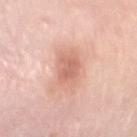biopsy status = catalogued during a skin exam; not biopsied | site = the chest | illumination = white-light | lesion size = ~4.5 mm (longest diameter) | automated lesion analysis = a lesion area of about 9.5 mm², a shape eccentricity near 0.8, and a symmetry-axis asymmetry near 0.2 | patient = female, approximately 65 years of age | image source = ~15 mm tile from a whole-body skin photo.